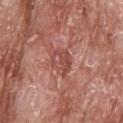TBP lesion metrics: a footprint of about 6 mm², a shape eccentricity near 0.6, and a symmetry-axis asymmetry near 0.35; an average lesion color of about L≈49 a*≈27 b*≈26 (CIELAB) and a normalized lesion–skin contrast near 6; a classifier nevus-likeness of about 0/100 | body site: the head or neck | lesion size: ≈3 mm | tile lighting: white-light illumination | image: total-body-photography crop, ~15 mm field of view | patient: male, approximately 65 years of age.